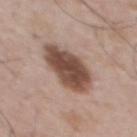Case summary:
* imaging modality: ~15 mm tile from a whole-body skin photo
* illumination: white-light
* automated metrics: an eccentricity of roughly 0.65 and a symmetry-axis asymmetry near 0.2; border irregularity of about 2 on a 0–10 scale, a color-variation rating of about 4.5/10, and peripheral color asymmetry of about 1.5
* subject: male, in their mid-50s
* site: the upper back